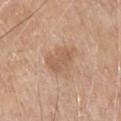Assessment: This lesion was catalogued during total-body skin photography and was not selected for biopsy. Acquisition and patient details: The lesion is located on the chest. About 4 mm across. A 15 mm close-up extracted from a 3D total-body photography capture. The tile uses white-light illumination. The subject is a male in their 80s.